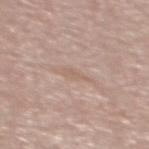Q: Was this lesion biopsied?
A: imaged on a skin check; not biopsied
Q: Illumination type?
A: white-light illumination
Q: What kind of image is this?
A: ~15 mm crop, total-body skin-cancer survey
Q: Lesion size?
A: ~2.5 mm (longest diameter)
Q: Where on the body is the lesion?
A: the upper back
Q: Patient demographics?
A: female, in their 50s